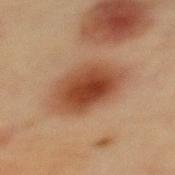follow-up: no biopsy performed (imaged during a skin exam); imaging modality: ~15 mm crop, total-body skin-cancer survey; patient: female, roughly 65 years of age; site: the upper back; size: ≈7 mm.